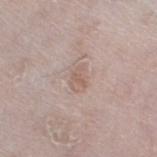notes: no biopsy performed (imaged during a skin exam) | illumination: white-light illumination | patient: male, aged around 65 | lesion size: ~2.5 mm (longest diameter) | body site: the left thigh | imaging modality: ~15 mm crop, total-body skin-cancer survey | TBP lesion metrics: an area of roughly 3.5 mm², an outline eccentricity of about 0.8 (0 = round, 1 = elongated), and two-axis asymmetry of about 0.3; border irregularity of about 3 on a 0–10 scale, a color-variation rating of about 1/10, and radial color variation of about 0.5.